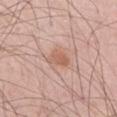The lesion was photographed on a routine skin check and not biopsied; there is no pathology result. Cropped from a whole-body photographic skin survey; the tile spans about 15 mm. This is a white-light tile. The lesion is on the right thigh. A male patient, in their mid-50s.